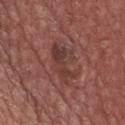| feature | finding |
|---|---|
| biopsy status | total-body-photography surveillance lesion; no biopsy |
| subject | male, aged 63–67 |
| lesion size | ~5 mm (longest diameter) |
| TBP lesion metrics | an area of roughly 9 mm², a shape eccentricity near 0.85, and two-axis asymmetry of about 0.45; border irregularity of about 5.5 on a 0–10 scale and a color-variation rating of about 4/10 |
| image source | total-body-photography crop, ~15 mm field of view |
| body site | the front of the torso |